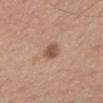- follow-up · catalogued during a skin exam; not biopsied
- illumination · white-light illumination
- anatomic site · the mid back
- diameter · about 2.5 mm
- subject · male, aged 53 to 57
- image source · total-body-photography crop, ~15 mm field of view
- automated metrics · an area of roughly 4.5 mm² and two-axis asymmetry of about 0.2; a border-irregularity rating of about 1.5/10